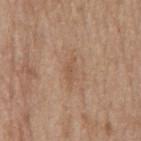| feature | finding |
|---|---|
| workup | total-body-photography surveillance lesion; no biopsy |
| tile lighting | white-light |
| patient | female, in their mid- to late 60s |
| location | the mid back |
| image source | 15 mm crop, total-body photography |
| image-analysis metrics | a footprint of about 3 mm², an eccentricity of roughly 0.95, and a shape-asymmetry score of about 0.35 (0 = symmetric); a border-irregularity rating of about 4/10 and peripheral color asymmetry of about 0; a nevus-likeness score of about 0/100 |
| size | ~3.5 mm (longest diameter) |